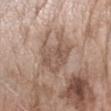This lesion was catalogued during total-body skin photography and was not selected for biopsy.
The lesion is on the right forearm.
A roughly 15 mm field-of-view crop from a total-body skin photograph.
A female patient in their mid-70s.